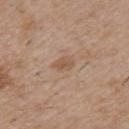Q: Was this lesion biopsied?
A: catalogued during a skin exam; not biopsied
Q: Where on the body is the lesion?
A: the chest
Q: Who is the patient?
A: male, aged 48 to 52
Q: What is the lesion's diameter?
A: ~2.5 mm (longest diameter)
Q: What is the imaging modality?
A: 15 mm crop, total-body photography
Q: What lighting was used for the tile?
A: white-light illumination
Q: What did automated image analysis measure?
A: a border-irregularity index near 2/10 and peripheral color asymmetry of about 1